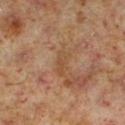Q: What is the imaging modality?
A: ~15 mm crop, total-body skin-cancer survey
Q: Illumination type?
A: cross-polarized
Q: Automated lesion metrics?
A: a lesion color around L≈45 a*≈18 b*≈32 in CIELAB, about 5 CIELAB-L* units darker than the surrounding skin, and a lesion-to-skin contrast of about 5.5 (normalized; higher = more distinct)
Q: Who is the patient?
A: male, roughly 60 years of age
Q: Where on the body is the lesion?
A: the leg
Q: Lesion size?
A: about 3 mm